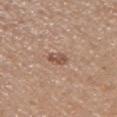{"biopsy_status": "not biopsied; imaged during a skin examination", "lighting": "white-light", "site": "right upper arm", "patient": {"sex": "male", "age_approx": 55}, "image": {"source": "total-body photography crop", "field_of_view_mm": 15}}